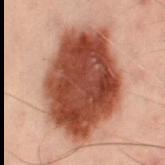Captured during whole-body skin photography for melanoma surveillance; the lesion was not biopsied. Located on the left thigh. The tile uses cross-polarized illumination. The total-body-photography lesion software estimated an eccentricity of roughly 0.8. It also reported border irregularity of about 2 on a 0–10 scale and peripheral color asymmetry of about 2. The patient is a male aged 48–52. Cropped from a total-body skin-imaging series; the visible field is about 15 mm.